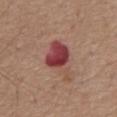Recorded during total-body skin imaging; not selected for excision or biopsy. This is a white-light tile. Automated tile analysis of the lesion measured a lesion color around L≈43 a*≈29 b*≈23 in CIELAB, a lesion–skin lightness drop of about 14, and a normalized border contrast of about 10.5. And it measured a border-irregularity rating of about 4.5/10, internal color variation of about 4.5 on a 0–10 scale, and a peripheral color-asymmetry measure near 1.5. The software also gave lesion-presence confidence of about 100/100. The lesion is on the abdomen. A male patient aged around 75. Measured at roughly 5 mm in maximum diameter. A region of skin cropped from a whole-body photographic capture, roughly 15 mm wide.